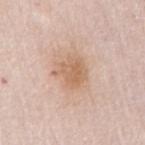{
  "biopsy_status": "not biopsied; imaged during a skin examination",
  "automated_metrics": {
    "area_mm2_approx": 8.0,
    "eccentricity": 0.5,
    "shape_asymmetry": 0.25
  },
  "lighting": "white-light",
  "lesion_size": {
    "long_diameter_mm_approx": 3.5
  },
  "site": "right upper arm",
  "image": {
    "source": "total-body photography crop",
    "field_of_view_mm": 15
  },
  "patient": {
    "sex": "male",
    "age_approx": 65
  }
}